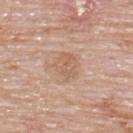{"biopsy_status": "not biopsied; imaged during a skin examination", "patient": {"sex": "male", "age_approx": 75}, "lighting": "white-light", "image": {"source": "total-body photography crop", "field_of_view_mm": 15}, "site": "back"}